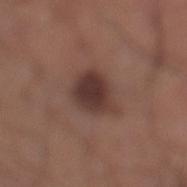The lesion was photographed on a routine skin check and not biopsied; there is no pathology result. The tile uses white-light illumination. Located on the right lower leg. Longest diameter approximately 5 mm. Cropped from a whole-body photographic skin survey; the tile spans about 15 mm. A male patient aged 43–47.